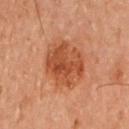Q: What is the lesion's diameter?
A: about 5 mm
Q: What kind of image is this?
A: ~15 mm tile from a whole-body skin photo
Q: Who is the patient?
A: female, roughly 60 years of age
Q: Lesion location?
A: the left arm
Q: What lighting was used for the tile?
A: cross-polarized